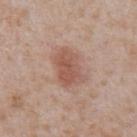Captured during whole-body skin photography for melanoma surveillance; the lesion was not biopsied. A lesion tile, about 15 mm wide, cut from a 3D total-body photograph. A male patient, aged approximately 65. Imaged with white-light lighting. The lesion-visualizer software estimated two-axis asymmetry of about 0.2. The analysis additionally found roughly 10 lightness units darker than nearby skin and a normalized lesion–skin contrast near 7. The lesion is on the abdomen.